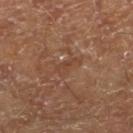No biopsy was performed on this lesion — it was imaged during a full skin examination and was not determined to be concerning. The lesion's longest dimension is about 3 mm. The lesion is located on the right lower leg. A male subject aged 68–72. This is a cross-polarized tile. Automated tile analysis of the lesion measured a lesion color around L≈41 a*≈20 b*≈29 in CIELAB, roughly 5 lightness units darker than nearby skin, and a normalized lesion–skin contrast near 4.5. It also reported border irregularity of about 6 on a 0–10 scale, a within-lesion color-variation index near 0.5/10, and a peripheral color-asymmetry measure near 0. The software also gave a nevus-likeness score of about 0/100 and a detector confidence of about 80 out of 100 that the crop contains a lesion. A roughly 15 mm field-of-view crop from a total-body skin photograph.